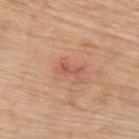<case>
  <site>upper back</site>
  <lesion_size>
    <long_diameter_mm_approx>3.0</long_diameter_mm_approx>
  </lesion_size>
  <automated_metrics>
    <eccentricity>0.9</eccentricity>
    <border_irregularity_0_10>5.5</border_irregularity_0_10>
    <color_variation_0_10>0.0</color_variation_0_10>
    <peripheral_color_asymmetry>0.0</peripheral_color_asymmetry>
    <nevus_likeness_0_100>0</nevus_likeness_0_100>
  </automated_metrics>
  <patient>
    <sex>female</sex>
    <age_approx>75</age_approx>
  </patient>
  <image>
    <source>total-body photography crop</source>
    <field_of_view_mm>15</field_of_view_mm>
  </image>
  <lighting>white-light</lighting>
</case>